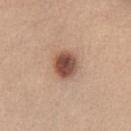follow-up: no biopsy performed (imaged during a skin exam) | subject: female, in their mid-40s | acquisition: ~15 mm tile from a whole-body skin photo | site: the chest | tile lighting: white-light illumination | image-analysis metrics: a footprint of about 8 mm², an eccentricity of roughly 0.55, and a shape-asymmetry score of about 0.1 (0 = symmetric); an average lesion color of about L≈50 a*≈21 b*≈28 (CIELAB) and roughly 17 lightness units darker than nearby skin; a border-irregularity rating of about 1/10 and a peripheral color-asymmetry measure near 1.5 | diameter: ≈3.5 mm.